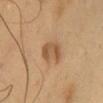Q: Is there a histopathology result?
A: no biopsy performed (imaged during a skin exam)
Q: What are the patient's age and sex?
A: male, in their 50s
Q: Lesion location?
A: the front of the torso
Q: What is the imaging modality?
A: 15 mm crop, total-body photography
Q: How was the tile lit?
A: cross-polarized
Q: Lesion size?
A: ≈3.5 mm